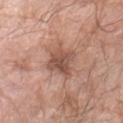The lesion was tiled from a total-body skin photograph and was not biopsied. A male patient, about 60 years old. Longest diameter approximately 4 mm. This image is a 15 mm lesion crop taken from a total-body photograph. Located on the left forearm. The tile uses white-light illumination.